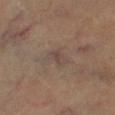Q: Was a biopsy performed?
A: catalogued during a skin exam; not biopsied
Q: What did automated image analysis measure?
A: a lesion area of about 4 mm², a shape eccentricity near 0.8, and a symmetry-axis asymmetry near 0.45; a border-irregularity index near 4/10 and internal color variation of about 2.5 on a 0–10 scale; an automated nevus-likeness rating near 0 out of 100 and a lesion-detection confidence of about 70/100
Q: What kind of image is this?
A: total-body-photography crop, ~15 mm field of view
Q: What is the lesion's diameter?
A: ≈3 mm
Q: Lesion location?
A: the right lower leg
Q: How was the tile lit?
A: cross-polarized
Q: Who is the patient?
A: male, approximately 60 years of age